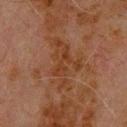follow-up: catalogued during a skin exam; not biopsied | anatomic site: the upper back | lesion diameter: ≈6.5 mm | tile lighting: cross-polarized | subject: male, aged 78 to 82 | automated lesion analysis: a lesion area of about 17 mm², an outline eccentricity of about 0.8 (0 = round, 1 = elongated), and a shape-asymmetry score of about 0.4 (0 = symmetric); a lesion color around L≈30 a*≈18 b*≈27 in CIELAB and a lesion–skin lightness drop of about 5; a border-irregularity rating of about 8/10, a color-variation rating of about 3/10, and peripheral color asymmetry of about 1 | acquisition: ~15 mm crop, total-body skin-cancer survey.